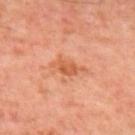{"biopsy_status": "not biopsied; imaged during a skin examination", "lesion_size": {"long_diameter_mm_approx": 3.5}, "image": {"source": "total-body photography crop", "field_of_view_mm": 15}, "patient": {"sex": "male", "age_approx": 50}, "site": "upper back"}